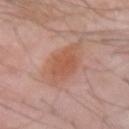biopsy status — catalogued during a skin exam; not biopsied | subject — male, aged approximately 65 | anatomic site — the right forearm | lighting — white-light illumination | image source — ~15 mm tile from a whole-body skin photo.